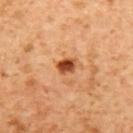biopsy status = imaged on a skin check; not biopsied | patient = female, roughly 55 years of age | anatomic site = the upper back | image source = ~15 mm tile from a whole-body skin photo.